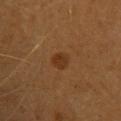Findings:
– follow-up — imaged on a skin check; not biopsied
– patient — female, in their mid-60s
– lesion size — ≈2 mm
– body site — the upper back
– image source — 15 mm crop, total-body photography
– tile lighting — cross-polarized